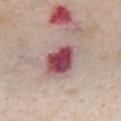Assessment:
This lesion was catalogued during total-body skin photography and was not selected for biopsy.
Context:
Cropped from a total-body skin-imaging series; the visible field is about 15 mm. The lesion is located on the front of the torso. A female patient, in their 40s. The total-body-photography lesion software estimated a lesion-detection confidence of about 100/100. The tile uses white-light illumination.